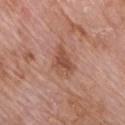Impression:
Captured during whole-body skin photography for melanoma surveillance; the lesion was not biopsied.
Clinical summary:
A lesion tile, about 15 mm wide, cut from a 3D total-body photograph. The patient is a male aged around 60. Imaged with white-light lighting. Automated tile analysis of the lesion measured an average lesion color of about L≈51 a*≈23 b*≈29 (CIELAB) and about 9 CIELAB-L* units darker than the surrounding skin. It also reported a border-irregularity index near 4.5/10, a within-lesion color-variation index near 3.5/10, and radial color variation of about 1.5. From the chest. About 3.5 mm across.